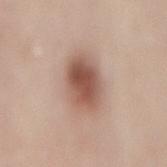Clinical impression:
Imaged during a routine full-body skin examination; the lesion was not biopsied and no histopathology is available.
Background:
Captured under white-light illumination. A 15 mm close-up extracted from a 3D total-body photography capture. The lesion is located on the mid back. Measured at roughly 5.5 mm in maximum diameter. The patient is a female in their 40s.